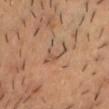follow-up = no biopsy performed (imaged during a skin exam)
image = 15 mm crop, total-body photography
location = the chest
patient = male, about 45 years old